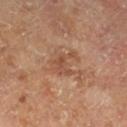Imaged during a routine full-body skin examination; the lesion was not biopsied and no histopathology is available. A lesion tile, about 15 mm wide, cut from a 3D total-body photograph. On the right lower leg. The subject is a male about 65 years old. Captured under cross-polarized illumination. Approximately 3 mm at its widest. Automated tile analysis of the lesion measured a nevus-likeness score of about 0/100.